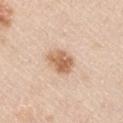Clinical impression:
Captured during whole-body skin photography for melanoma surveillance; the lesion was not biopsied.
Acquisition and patient details:
An algorithmic analysis of the crop reported a footprint of about 8 mm², a shape eccentricity near 0.65, and a symmetry-axis asymmetry near 0.25. It also reported a mean CIELAB color near L≈64 a*≈20 b*≈34 and a lesion–skin lightness drop of about 13. The analysis additionally found lesion-presence confidence of about 100/100. On the left upper arm. A male patient in their mid-40s. The recorded lesion diameter is about 3.5 mm. A 15 mm close-up extracted from a 3D total-body photography capture.